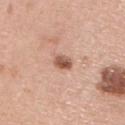Captured under white-light illumination.
Located on the right upper arm.
An algorithmic analysis of the crop reported an average lesion color of about L≈56 a*≈23 b*≈30 (CIELAB). The software also gave a border-irregularity index near 2.5/10, a within-lesion color-variation index near 5/10, and radial color variation of about 2.
A female patient aged around 60.
A lesion tile, about 15 mm wide, cut from a 3D total-body photograph.
The lesion's longest dimension is about 2.5 mm.